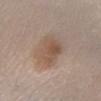Clinical summary: On the right lower leg. Automated image analysis of the tile measured an area of roughly 12 mm², an eccentricity of roughly 0.7, and two-axis asymmetry of about 0.15. It also reported a border-irregularity index near 2/10. The software also gave a detector confidence of about 100 out of 100 that the crop contains a lesion. Imaged with white-light lighting. A male patient aged approximately 65. Cropped from a whole-body photographic skin survey; the tile spans about 15 mm. About 4.5 mm across.